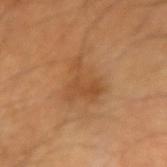Q: Was this lesion biopsied?
A: no biopsy performed (imaged during a skin exam)
Q: What is the imaging modality?
A: ~15 mm crop, total-body skin-cancer survey
Q: Lesion location?
A: the right forearm
Q: Patient demographics?
A: male, aged 58 to 62
Q: How was the tile lit?
A: cross-polarized illumination
Q: How large is the lesion?
A: ≈4.5 mm
Q: Automated lesion metrics?
A: an area of roughly 10 mm² and an eccentricity of roughly 0.65; a lesion–skin lightness drop of about 7 and a lesion-to-skin contrast of about 5.5 (normalized; higher = more distinct); a border-irregularity rating of about 7/10, a within-lesion color-variation index near 2.5/10, and a peripheral color-asymmetry measure near 1